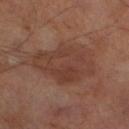The lesion was photographed on a routine skin check and not biopsied; there is no pathology result.
From the right thigh.
The total-body-photography lesion software estimated a mean CIELAB color near L≈38 a*≈20 b*≈25 and a lesion–skin lightness drop of about 7. The analysis additionally found a detector confidence of about 100 out of 100 that the crop contains a lesion.
A male subject, about 70 years old.
The recorded lesion diameter is about 7.5 mm.
A 15 mm close-up tile from a total-body photography series done for melanoma screening.